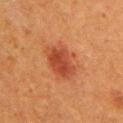Part of a total-body skin-imaging series; this lesion was reviewed on a skin check and was not flagged for biopsy. The lesion-visualizer software estimated a footprint of about 12 mm², an outline eccentricity of about 0.5 (0 = round, 1 = elongated), and a symmetry-axis asymmetry near 0.2. And it measured a lesion color around L≈39 a*≈27 b*≈32 in CIELAB, a lesion–skin lightness drop of about 9, and a normalized border contrast of about 7.5. The software also gave internal color variation of about 3 on a 0–10 scale and a peripheral color-asymmetry measure near 1. And it measured a classifier nevus-likeness of about 95/100 and a lesion-detection confidence of about 100/100. On the right upper arm. Imaged with cross-polarized lighting. A 15 mm close-up tile from a total-body photography series done for melanoma screening. A female subject, roughly 40 years of age. About 4.5 mm across.